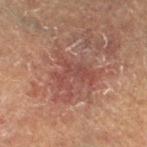Assessment: Recorded during total-body skin imaging; not selected for excision or biopsy. Background: The patient is a male aged around 65. Located on the left lower leg. A 15 mm crop from a total-body photograph taken for skin-cancer surveillance. Imaged with cross-polarized lighting. Measured at roughly 5 mm in maximum diameter.